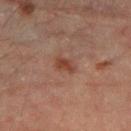No biopsy was performed on this lesion — it was imaged during a full skin examination and was not determined to be concerning. Measured at roughly 2.5 mm in maximum diameter. The tile uses cross-polarized illumination. A male patient, roughly 45 years of age. The lesion is located on the left forearm. The lesion-visualizer software estimated a footprint of about 3.5 mm², an eccentricity of roughly 0.8, and two-axis asymmetry of about 0.35. And it measured a mean CIELAB color near L≈36 a*≈20 b*≈25, about 8 CIELAB-L* units darker than the surrounding skin, and a normalized lesion–skin contrast near 7.5. And it measured a nevus-likeness score of about 30/100 and a detector confidence of about 100 out of 100 that the crop contains a lesion. Cropped from a total-body skin-imaging series; the visible field is about 15 mm.